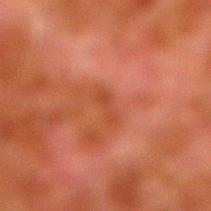biopsy status = total-body-photography surveillance lesion; no biopsy | anatomic site = the right lower leg | lesion size = ~3 mm (longest diameter) | image = 15 mm crop, total-body photography | subject = male, aged 78–82.